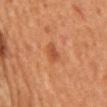Imaged during a routine full-body skin examination; the lesion was not biopsied and no histopathology is available.
This image is a 15 mm lesion crop taken from a total-body photograph.
The lesion is located on the chest.
The subject is a male aged 58 to 62.